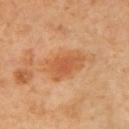Clinical impression:
Recorded during total-body skin imaging; not selected for excision or biopsy.
Image and clinical context:
The total-body-photography lesion software estimated a footprint of about 13 mm² and a shape-asymmetry score of about 0.25 (0 = symmetric). The software also gave an average lesion color of about L≈59 a*≈26 b*≈42 (CIELAB) and about 8 CIELAB-L* units darker than the surrounding skin. And it measured border irregularity of about 3 on a 0–10 scale, a color-variation rating of about 3/10, and peripheral color asymmetry of about 1. And it measured a classifier nevus-likeness of about 80/100. This is a cross-polarized tile. Longest diameter approximately 5 mm. Cropped from a total-body skin-imaging series; the visible field is about 15 mm. A female subject, approximately 55 years of age. The lesion is on the arm.